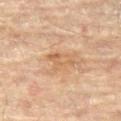Findings:
- follow-up: catalogued during a skin exam; not biopsied
- diameter: ~3.5 mm (longest diameter)
- subject: female, aged approximately 80
- location: the right leg
- imaging modality: ~15 mm crop, total-body skin-cancer survey
- tile lighting: cross-polarized illumination